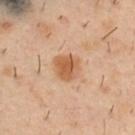Assessment:
Part of a total-body skin-imaging series; this lesion was reviewed on a skin check and was not flagged for biopsy.
Background:
This image is a 15 mm lesion crop taken from a total-body photograph. A male subject, aged 38 to 42. Captured under cross-polarized illumination. On the upper back.